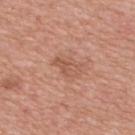* follow-up: imaged on a skin check; not biopsied
* site: the upper back
* subject: female, aged 38 to 42
* image-analysis metrics: roughly 7 lightness units darker than nearby skin; a classifier nevus-likeness of about 0/100 and lesion-presence confidence of about 100/100
* acquisition: ~15 mm crop, total-body skin-cancer survey